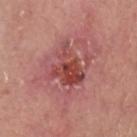A lesion tile, about 15 mm wide, cut from a 3D total-body photograph. Located on the head or neck. A male patient aged 63 to 67. Histopathologically confirmed as a skin cancer: squamous cell carcinoma in situ.